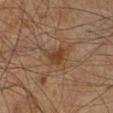Part of a total-body skin-imaging series; this lesion was reviewed on a skin check and was not flagged for biopsy. A roughly 15 mm field-of-view crop from a total-body skin photograph. Located on the left lower leg. Longest diameter approximately 3 mm. The total-body-photography lesion software estimated a lesion–skin lightness drop of about 8 and a lesion-to-skin contrast of about 7.5 (normalized; higher = more distinct). A male subject roughly 60 years of age.